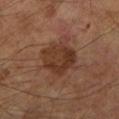Imaged during a routine full-body skin examination; the lesion was not biopsied and no histopathology is available.
The subject is a male aged approximately 65.
A 15 mm close-up extracted from a 3D total-body photography capture.
The total-body-photography lesion software estimated an eccentricity of roughly 0.45. The analysis additionally found a lesion color around L≈34 a*≈20 b*≈27 in CIELAB and a normalized border contrast of about 8. The analysis additionally found a border-irregularity rating of about 2.5/10, internal color variation of about 4 on a 0–10 scale, and a peripheral color-asymmetry measure near 1.5.
This is a cross-polarized tile.
Approximately 5 mm at its widest.